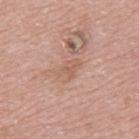notes: no biopsy performed (imaged during a skin exam)
automated metrics: border irregularity of about 4 on a 0–10 scale, a within-lesion color-variation index near 2/10, and a peripheral color-asymmetry measure near 0.5; a nevus-likeness score of about 0/100 and lesion-presence confidence of about 80/100
patient: male, aged 68 to 72
imaging modality: ~15 mm tile from a whole-body skin photo
site: the upper back
illumination: white-light illumination
diameter: ~3.5 mm (longest diameter)